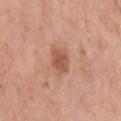{
  "biopsy_status": "not biopsied; imaged during a skin examination",
  "automated_metrics": {
    "cielab_L": 54,
    "cielab_a": 23,
    "cielab_b": 31,
    "vs_skin_darker_L": 11.0,
    "vs_skin_contrast_norm": 7.5
  },
  "patient": {
    "sex": "female",
    "age_approx": 65
  },
  "lesion_size": {
    "long_diameter_mm_approx": 2.5
  },
  "site": "left upper arm",
  "image": {
    "source": "total-body photography crop",
    "field_of_view_mm": 15
  },
  "lighting": "white-light"
}